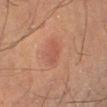  biopsy_status: not biopsied; imaged during a skin examination
  lesion_size:
    long_diameter_mm_approx: 3.5
  image:
    source: total-body photography crop
    field_of_view_mm: 15
  automated_metrics:
    cielab_L: 46
    cielab_a: 23
    cielab_b: 27
    vs_skin_contrast_norm: 5.0
    nevus_likeness_0_100: 45
  lighting: cross-polarized
  patient:
    sex: male
    age_approx: 60